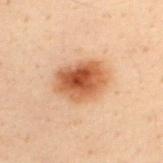| key | value |
|---|---|
| location | the upper back |
| lesion size | about 5.5 mm |
| automated lesion analysis | an average lesion color of about L≈47 a*≈21 b*≈32 (CIELAB), roughly 13 lightness units darker than nearby skin, and a normalized lesion–skin contrast near 10; a border-irregularity rating of about 1.5/10, a within-lesion color-variation index near 7/10, and radial color variation of about 2 |
| patient | male, aged approximately 30 |
| illumination | cross-polarized illumination |
| imaging modality | ~15 mm tile from a whole-body skin photo |